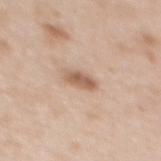Assessment:
The lesion was photographed on a routine skin check and not biopsied; there is no pathology result.
Clinical summary:
About 3 mm across. From the mid back. This image is a 15 mm lesion crop taken from a total-body photograph. The subject is a female in their 50s.